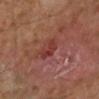Clinical impression: No biopsy was performed on this lesion — it was imaged during a full skin examination and was not determined to be concerning. Background: The lesion is located on the right lower leg. Automated image analysis of the tile measured roughly 7 lightness units darker than nearby skin and a normalized border contrast of about 6.5. The software also gave a border-irregularity rating of about 4/10, a within-lesion color-variation index near 2.5/10, and a peripheral color-asymmetry measure near 0.5. Imaged with cross-polarized lighting. A male patient about 60 years old. This image is a 15 mm lesion crop taken from a total-body photograph.